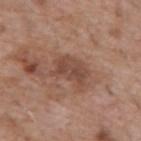biopsy status=no biopsy performed (imaged during a skin exam) | illumination=white-light | imaging modality=15 mm crop, total-body photography | patient=male, aged 58–62 | diameter=≈4 mm | location=the chest.